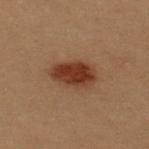Imaged during a routine full-body skin examination; the lesion was not biopsied and no histopathology is available.
On the upper back.
A female subject aged around 30.
The tile uses cross-polarized illumination.
A close-up tile cropped from a whole-body skin photograph, about 15 mm across.